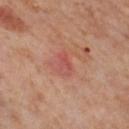No biopsy was performed on this lesion — it was imaged during a full skin examination and was not determined to be concerning. Longest diameter approximately 2.5 mm. From the left thigh. A female patient approximately 55 years of age. Cropped from a whole-body photographic skin survey; the tile spans about 15 mm.